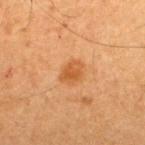Q: Was a biopsy performed?
A: no biopsy performed (imaged during a skin exam)
Q: Patient demographics?
A: male, roughly 65 years of age
Q: How was this image acquired?
A: ~15 mm tile from a whole-body skin photo
Q: What is the lesion's diameter?
A: ≈3 mm
Q: What did automated image analysis measure?
A: a lesion area of about 5 mm², an eccentricity of roughly 0.65, and two-axis asymmetry of about 0.25; a classifier nevus-likeness of about 85/100 and a lesion-detection confidence of about 100/100
Q: Where on the body is the lesion?
A: the upper back
Q: How was the tile lit?
A: cross-polarized